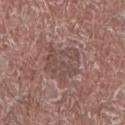biopsy status: catalogued during a skin exam; not biopsied
diameter: ≈4.5 mm
illumination: white-light illumination
anatomic site: the right forearm
imaging modality: ~15 mm tile from a whole-body skin photo
subject: male, aged 68–72